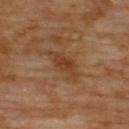Captured during whole-body skin photography for melanoma surveillance; the lesion was not biopsied.
A male subject approximately 70 years of age.
The lesion is on the upper back.
Cropped from a whole-body photographic skin survey; the tile spans about 15 mm.